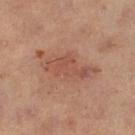Q: Was a biopsy performed?
A: catalogued during a skin exam; not biopsied
Q: What are the patient's age and sex?
A: female, aged approximately 40
Q: What is the anatomic site?
A: the left lower leg
Q: Automated lesion metrics?
A: border irregularity of about 4 on a 0–10 scale, a color-variation rating of about 3.5/10, and peripheral color asymmetry of about 1.5; a classifier nevus-likeness of about 0/100 and lesion-presence confidence of about 100/100
Q: What lighting was used for the tile?
A: cross-polarized illumination
Q: How large is the lesion?
A: about 6.5 mm
Q: What is the imaging modality?
A: total-body-photography crop, ~15 mm field of view